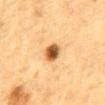Clinical impression:
Captured during whole-body skin photography for melanoma surveillance; the lesion was not biopsied.
Acquisition and patient details:
The lesion's longest dimension is about 3 mm. The tile uses cross-polarized illumination. A lesion tile, about 15 mm wide, cut from a 3D total-body photograph. From the front of the torso. A male subject, aged 83 or older. An algorithmic analysis of the crop reported a border-irregularity index near 1.5/10, internal color variation of about 7.5 on a 0–10 scale, and radial color variation of about 2.5. The analysis additionally found a classifier nevus-likeness of about 100/100.